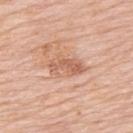Case summary:
- follow-up — no biopsy performed (imaged during a skin exam)
- automated metrics — a normalized lesion–skin contrast near 6.5; an automated nevus-likeness rating near 10 out of 100 and a detector confidence of about 100 out of 100 that the crop contains a lesion
- size — ≈4.5 mm
- subject — male, aged 78–82
- location — the upper back
- image — 15 mm crop, total-body photography
- lighting — white-light